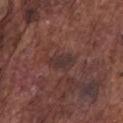Q: What kind of image is this?
A: ~15 mm crop, total-body skin-cancer survey
Q: Lesion location?
A: the chest
Q: Automated lesion metrics?
A: a footprint of about 6.5 mm² and a symmetry-axis asymmetry near 0.2; a within-lesion color-variation index near 2/10 and a peripheral color-asymmetry measure near 1; an automated nevus-likeness rating near 0 out of 100 and lesion-presence confidence of about 90/100
Q: How large is the lesion?
A: ≈4 mm
Q: What are the patient's age and sex?
A: male, approximately 75 years of age
Q: What lighting was used for the tile?
A: white-light